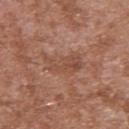{"biopsy_status": "not biopsied; imaged during a skin examination", "site": "upper back", "patient": {"sex": "male", "age_approx": 45}, "image": {"source": "total-body photography crop", "field_of_view_mm": 15}}